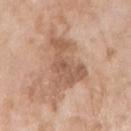Impression:
Captured during whole-body skin photography for melanoma surveillance; the lesion was not biopsied.
Acquisition and patient details:
The lesion is located on the right upper arm. The patient is a female in their mid- to late 70s. A 15 mm crop from a total-body photograph taken for skin-cancer surveillance.